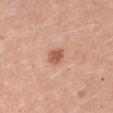Notes:
- notes: no biopsy performed (imaged during a skin exam)
- tile lighting: white-light illumination
- anatomic site: the right upper arm
- image source: 15 mm crop, total-body photography
- patient: male, aged around 25
- TBP lesion metrics: an area of roughly 4 mm², a shape eccentricity near 0.65, and a symmetry-axis asymmetry near 0.25; about 12 CIELAB-L* units darker than the surrounding skin and a lesion-to-skin contrast of about 8 (normalized; higher = more distinct); border irregularity of about 2 on a 0–10 scale and peripheral color asymmetry of about 1; a lesion-detection confidence of about 100/100